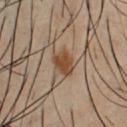  biopsy_status: not biopsied; imaged during a skin examination
  lesion_size:
    long_diameter_mm_approx: 3.0
  site: chest
  lighting: cross-polarized
  patient:
    sex: male
    age_approx: 40
  image:
    source: total-body photography crop
    field_of_view_mm: 15
  automated_metrics:
    area_mm2_approx: 5.5
    eccentricity: 0.7
    shape_asymmetry: 0.25
    cielab_L: 44
    cielab_a: 18
    cielab_b: 31
    vs_skin_darker_L: 11.0
    vs_skin_contrast_norm: 10.0
    nevus_likeness_0_100: 95
    lesion_detection_confidence_0_100: 100